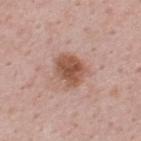No biopsy was performed on this lesion — it was imaged during a full skin examination and was not determined to be concerning.
Approximately 4 mm at its widest.
Imaged with white-light lighting.
The subject is a male approximately 50 years of age.
A region of skin cropped from a whole-body photographic capture, roughly 15 mm wide.
From the upper back.